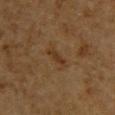The lesion is located on the upper back. A male subject, aged approximately 85 or older. A 15 mm close-up tile from a total-body photography series done for melanoma screening. About 3 mm across. Automated image analysis of the tile measured an average lesion color of about L≈29 a*≈15 b*≈29 (CIELAB) and a lesion-to-skin contrast of about 7 (normalized; higher = more distinct). The analysis additionally found a classifier nevus-likeness of about 0/100 and lesion-presence confidence of about 95/100. The tile uses cross-polarized illumination.